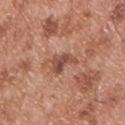notes: no biopsy performed (imaged during a skin exam)
acquisition: 15 mm crop, total-body photography
illumination: white-light
patient: male, aged around 55
TBP lesion metrics: a mean CIELAB color near L≈49 a*≈23 b*≈29 and a lesion-to-skin contrast of about 8 (normalized; higher = more distinct); a border-irregularity rating of about 5/10, a within-lesion color-variation index near 4/10, and radial color variation of about 1.5; a nevus-likeness score of about 0/100 and a detector confidence of about 100 out of 100 that the crop contains a lesion
body site: the upper back
lesion size: about 3.5 mm